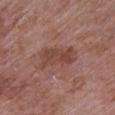biopsy_status: not biopsied; imaged during a skin examination
site: right upper arm
automated_metrics:
  eccentricity: 0.85
  shape_asymmetry: 0.3
  cielab_L: 44
  cielab_a: 21
  cielab_b: 25
  vs_skin_contrast_norm: 6.5
  border_irregularity_0_10: 4.0
  color_variation_0_10: 2.5
  peripheral_color_asymmetry: 1.0
  nevus_likeness_0_100: 0
  lesion_detection_confidence_0_100: 100
patient:
  sex: female
  age_approx: 70
lesion_size:
  long_diameter_mm_approx: 5.0
lighting: white-light
image:
  source: total-body photography crop
  field_of_view_mm: 15